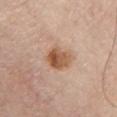The lesion was photographed on a routine skin check and not biopsied; there is no pathology result. The recorded lesion diameter is about 3.5 mm. The lesion is on the chest. The lesion-visualizer software estimated a mean CIELAB color near L≈56 a*≈21 b*≈33 and a normalized border contrast of about 9. It also reported a within-lesion color-variation index near 7/10 and peripheral color asymmetry of about 2.5. This is a white-light tile. A 15 mm close-up extracted from a 3D total-body photography capture. The patient is a male roughly 70 years of age.